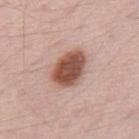Impression:
This lesion was catalogued during total-body skin photography and was not selected for biopsy.
Background:
The lesion's longest dimension is about 4.5 mm. Cropped from a total-body skin-imaging series; the visible field is about 15 mm. The tile uses white-light illumination. A male subject, roughly 70 years of age. The lesion is on the mid back.